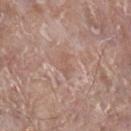| field | value |
|---|---|
| workup | total-body-photography surveillance lesion; no biopsy |
| automated lesion analysis | a border-irregularity rating of about 4/10 and radial color variation of about 0; an automated nevus-likeness rating near 0 out of 100 and a detector confidence of about 85 out of 100 that the crop contains a lesion |
| body site | the right lower leg |
| subject | male, approximately 45 years of age |
| size | about 2.5 mm |
| acquisition | ~15 mm crop, total-body skin-cancer survey |